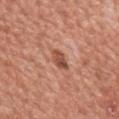Q: Is there a histopathology result?
A: no biopsy performed (imaged during a skin exam)
Q: What is the lesion's diameter?
A: ~2.5 mm (longest diameter)
Q: What is the anatomic site?
A: the chest
Q: What did automated image analysis measure?
A: an average lesion color of about L≈51 a*≈26 b*≈30 (CIELAB), a lesion–skin lightness drop of about 12, and a normalized lesion–skin contrast near 8; a border-irregularity rating of about 2/10, internal color variation of about 4 on a 0–10 scale, and radial color variation of about 1.5
Q: How was the tile lit?
A: white-light illumination
Q: How was this image acquired?
A: 15 mm crop, total-body photography
Q: Patient demographics?
A: male, in their mid-40s